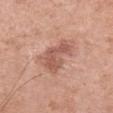Case summary:
- notes · imaged on a skin check; not biopsied
- diameter · ~4.5 mm (longest diameter)
- image-analysis metrics · a footprint of about 9 mm² and a shape-asymmetry score of about 0.4 (0 = symmetric); a border-irregularity index near 5.5/10, a color-variation rating of about 2.5/10, and peripheral color asymmetry of about 1; a nevus-likeness score of about 5/100 and lesion-presence confidence of about 100/100
- image source · 15 mm crop, total-body photography
- site · the left upper arm
- subject · female, about 70 years old
- lighting · white-light